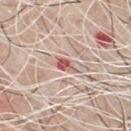follow-up: catalogued during a skin exam; not biopsied | imaging modality: ~15 mm crop, total-body skin-cancer survey | patient: male, approximately 70 years of age | anatomic site: the chest.